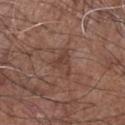Assessment: The lesion was tiled from a total-body skin photograph and was not biopsied. Background: The tile uses white-light illumination. A 15 mm close-up extracted from a 3D total-body photography capture. From the arm. Measured at roughly 3.5 mm in maximum diameter. An algorithmic analysis of the crop reported a footprint of about 5.5 mm² and an eccentricity of roughly 0.7. The analysis additionally found a normalized lesion–skin contrast near 5.5. The analysis additionally found a within-lesion color-variation index near 3/10 and radial color variation of about 1.5. The software also gave lesion-presence confidence of about 95/100. A male subject, aged 73–77.